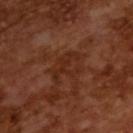Notes:
* imaging modality: ~15 mm crop, total-body skin-cancer survey
* patient: male, aged approximately 65
* diameter: ≈4 mm